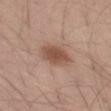Impression:
Imaged during a routine full-body skin examination; the lesion was not biopsied and no histopathology is available.
Context:
A 15 mm close-up extracted from a 3D total-body photography capture. From the left thigh. This is a white-light tile. The subject is a male aged around 30. Longest diameter approximately 4.5 mm.